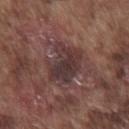image — ~15 mm tile from a whole-body skin photo; tile lighting — white-light; location — the chest; patient — male, in their mid-70s.